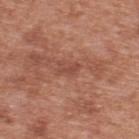<tbp_lesion>
  <biopsy_status>not biopsied; imaged during a skin examination</biopsy_status>
</tbp_lesion>